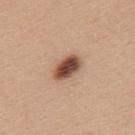Findings:
– follow-up: total-body-photography surveillance lesion; no biopsy